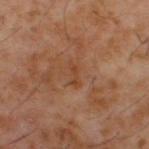| field | value |
|---|---|
| biopsy status | no biopsy performed (imaged during a skin exam) |
| acquisition | 15 mm crop, total-body photography |
| TBP lesion metrics | an area of roughly 2.5 mm², an outline eccentricity of about 0.9 (0 = round, 1 = elongated), and a shape-asymmetry score of about 0.45 (0 = symmetric); a border-irregularity index near 5/10 and a within-lesion color-variation index near 0/10 |
| anatomic site | the back |
| subject | male, aged approximately 60 |